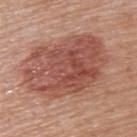{
  "biopsy_status": "not biopsied; imaged during a skin examination",
  "lesion_size": {
    "long_diameter_mm_approx": 11.0
  },
  "image": {
    "source": "total-body photography crop",
    "field_of_view_mm": 15
  },
  "lighting": "white-light",
  "site": "back",
  "patient": {
    "sex": "female",
    "age_approx": 50
  }
}